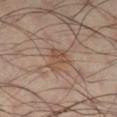The lesion was photographed on a routine skin check and not biopsied; there is no pathology result.
A lesion tile, about 15 mm wide, cut from a 3D total-body photograph.
Located on the leg.
The subject is a male aged 43–47.